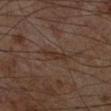Assessment: This lesion was catalogued during total-body skin photography and was not selected for biopsy. Context: Measured at roughly 3 mm in maximum diameter. On the right lower leg. A male subject, about 60 years old. A lesion tile, about 15 mm wide, cut from a 3D total-body photograph. This is a cross-polarized tile.